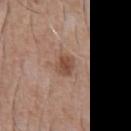| field | value |
|---|---|
| anatomic site | the abdomen |
| patient | male, aged approximately 55 |
| automated metrics | a mean CIELAB color near L≈48 a*≈20 b*≈28, about 10 CIELAB-L* units darker than the surrounding skin, and a lesion-to-skin contrast of about 8 (normalized; higher = more distinct); a border-irregularity rating of about 2/10, a color-variation rating of about 2.5/10, and radial color variation of about 1 |
| image source | ~15 mm tile from a whole-body skin photo |